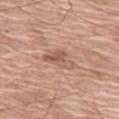biopsy_status: not biopsied; imaged during a skin examination
patient:
  sex: female
  age_approx: 75
site: leg
image:
  source: total-body photography crop
  field_of_view_mm: 15
lighting: white-light